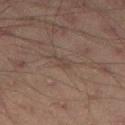Assessment: The lesion was tiled from a total-body skin photograph and was not biopsied. Image and clinical context: An algorithmic analysis of the crop reported a lesion color around L≈34 a*≈12 b*≈19 in CIELAB, roughly 4 lightness units darker than nearby skin, and a lesion-to-skin contrast of about 4.5 (normalized; higher = more distinct). The software also gave a border-irregularity rating of about 5/10 and a within-lesion color-variation index near 0.5/10. It also reported a classifier nevus-likeness of about 0/100 and lesion-presence confidence of about 75/100. A roughly 15 mm field-of-view crop from a total-body skin photograph. The lesion is located on the left thigh. The patient is a male aged approximately 45.